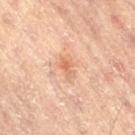The lesion was photographed on a routine skin check and not biopsied; there is no pathology result.
This is a cross-polarized tile.
Longest diameter approximately 2.5 mm.
Automated tile analysis of the lesion measured an area of roughly 3 mm², an eccentricity of roughly 0.85, and a shape-asymmetry score of about 0.35 (0 = symmetric). It also reported a mean CIELAB color near L≈60 a*≈21 b*≈33, roughly 8 lightness units darker than nearby skin, and a lesion-to-skin contrast of about 6 (normalized; higher = more distinct). And it measured peripheral color asymmetry of about 0. And it measured a classifier nevus-likeness of about 0/100 and lesion-presence confidence of about 100/100.
Cropped from a total-body skin-imaging series; the visible field is about 15 mm.
The lesion is located on the left leg.
A female subject in their 80s.